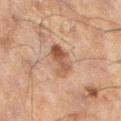Q: Is there a histopathology result?
A: imaged on a skin check; not biopsied
Q: What lighting was used for the tile?
A: cross-polarized illumination
Q: How was this image acquired?
A: ~15 mm tile from a whole-body skin photo
Q: Who is the patient?
A: male, aged 58–62
Q: What is the lesion's diameter?
A: ≈3.5 mm
Q: What is the anatomic site?
A: the left lower leg